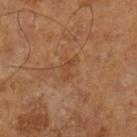<case>
  <biopsy_status>not biopsied; imaged during a skin examination</biopsy_status>
</case>